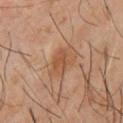Impression:
Captured during whole-body skin photography for melanoma surveillance; the lesion was not biopsied.
Background:
The lesion is located on the front of the torso. A male patient approximately 60 years of age. Measured at roughly 3 mm in maximum diameter. The tile uses cross-polarized illumination. A close-up tile cropped from a whole-body skin photograph, about 15 mm across.